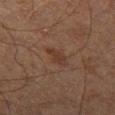Context:
A 15 mm close-up extracted from a 3D total-body photography capture. The recorded lesion diameter is about 3 mm. The total-body-photography lesion software estimated a footprint of about 4 mm², a shape eccentricity near 0.8, and a symmetry-axis asymmetry near 0.25. The software also gave border irregularity of about 3 on a 0–10 scale and radial color variation of about 0.5. The analysis additionally found a classifier nevus-likeness of about 0/100. A male patient about 65 years old. Imaged with cross-polarized lighting. Located on the left thigh.